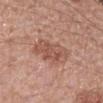follow-up = no biopsy performed (imaged during a skin exam)
image-analysis metrics = a mean CIELAB color near L≈53 a*≈23 b*≈28 and a normalized border contrast of about 6; border irregularity of about 3 on a 0–10 scale, internal color variation of about 3.5 on a 0–10 scale, and radial color variation of about 1
patient = female, about 50 years old
body site = the left forearm
image = ~15 mm crop, total-body skin-cancer survey
tile lighting = white-light
lesion size = ~4 mm (longest diameter)